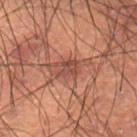Clinical impression: Imaged during a routine full-body skin examination; the lesion was not biopsied and no histopathology is available. Context: The subject is a male aged around 50. On the lower back. Automated image analysis of the tile measured a symmetry-axis asymmetry near 0.3. This image is a 15 mm lesion crop taken from a total-body photograph.